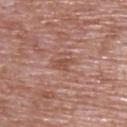Q: Is there a histopathology result?
A: no biopsy performed (imaged during a skin exam)
Q: What kind of image is this?
A: ~15 mm tile from a whole-body skin photo
Q: What did automated image analysis measure?
A: a footprint of about 3 mm² and a symmetry-axis asymmetry near 0.35; border irregularity of about 3.5 on a 0–10 scale, internal color variation of about 1 on a 0–10 scale, and radial color variation of about 0.5; a nevus-likeness score of about 0/100 and a detector confidence of about 95 out of 100 that the crop contains a lesion
Q: Lesion location?
A: the back
Q: How large is the lesion?
A: ~3 mm (longest diameter)
Q: What lighting was used for the tile?
A: white-light
Q: What are the patient's age and sex?
A: male, about 70 years old